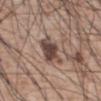{
  "biopsy_status": "not biopsied; imaged during a skin examination",
  "site": "abdomen",
  "image": {
    "source": "total-body photography crop",
    "field_of_view_mm": 15
  },
  "patient": {
    "sex": "male",
    "age_approx": 60
  },
  "lighting": "white-light",
  "lesion_size": {
    "long_diameter_mm_approx": 4.0
  }
}